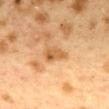Findings:
– biopsy status · total-body-photography surveillance lesion; no biopsy
– acquisition · total-body-photography crop, ~15 mm field of view
– anatomic site · the mid back
– patient · female, in their 40s